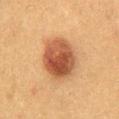Part of a total-body skin-imaging series; this lesion was reviewed on a skin check and was not flagged for biopsy.
The lesion is on the chest.
Longest diameter approximately 5 mm.
Cropped from a total-body skin-imaging series; the visible field is about 15 mm.
The tile uses cross-polarized illumination.
A male subject, aged approximately 50.